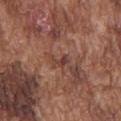This lesion was catalogued during total-body skin photography and was not selected for biopsy. Measured at roughly 2.5 mm in maximum diameter. A 15 mm close-up tile from a total-body photography series done for melanoma screening. The patient is a male aged 73–77. On the chest. Captured under white-light illumination.